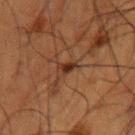  lighting: cross-polarized
  image:
    source: total-body photography crop
    field_of_view_mm: 15
  automated_metrics:
    border_irregularity_0_10: 3.5
    color_variation_0_10: 1.5
    peripheral_color_asymmetry: 0.5
  site: left upper arm
  lesion_size:
    long_diameter_mm_approx: 2.5
  patient:
    sex: male
    age_approx: 50